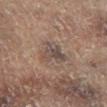On the leg. This is a cross-polarized tile. A region of skin cropped from a whole-body photographic capture, roughly 15 mm wide. About 3.5 mm across. The lesion-visualizer software estimated a footprint of about 7.5 mm², an eccentricity of roughly 0.5, and a shape-asymmetry score of about 0.5 (0 = symmetric). And it measured a lesion color around L≈44 a*≈13 b*≈20 in CIELAB, a lesion–skin lightness drop of about 8, and a normalized border contrast of about 7. The patient is a male approximately 65 years of age.